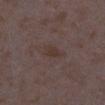The lesion was tiled from a total-body skin photograph and was not biopsied.
On the left lower leg.
The subject is a female aged 33–37.
A 15 mm close-up extracted from a 3D total-body photography capture.